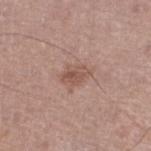The lesion was photographed on a routine skin check and not biopsied; there is no pathology result. A male patient in their mid-60s. The total-body-photography lesion software estimated border irregularity of about 3 on a 0–10 scale and internal color variation of about 4 on a 0–10 scale. And it measured a nevus-likeness score of about 10/100. A lesion tile, about 15 mm wide, cut from a 3D total-body photograph. Located on the leg. Measured at roughly 3.5 mm in maximum diameter.